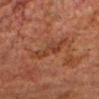Case summary:
• notes: total-body-photography surveillance lesion; no biopsy
• lesion diameter: ≈5 mm
• image: 15 mm crop, total-body photography
• site: the head or neck
• image-analysis metrics: an area of roughly 7.5 mm², an eccentricity of roughly 0.9, and two-axis asymmetry of about 0.35; a mean CIELAB color near L≈30 a*≈20 b*≈25, about 5 CIELAB-L* units darker than the surrounding skin, and a normalized border contrast of about 5.5; a border-irregularity index near 5.5/10, a color-variation rating of about 3/10, and radial color variation of about 1; an automated nevus-likeness rating near 0 out of 100
• patient: male, roughly 60 years of age
• tile lighting: cross-polarized illumination